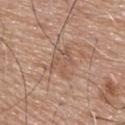Imaged during a routine full-body skin examination; the lesion was not biopsied and no histopathology is available. A lesion tile, about 15 mm wide, cut from a 3D total-body photograph. Approximately 4 mm at its widest. A male subject, aged around 75. On the upper back.